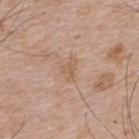Assessment: Captured during whole-body skin photography for melanoma surveillance; the lesion was not biopsied. Background: From the upper back. A male patient approximately 65 years of age. This image is a 15 mm lesion crop taken from a total-body photograph. The lesion-visualizer software estimated a border-irregularity rating of about 4.5/10, a color-variation rating of about 1/10, and a peripheral color-asymmetry measure near 0.5. Approximately 2.5 mm at its widest. This is a white-light tile.